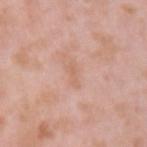| feature | finding |
|---|---|
| workup | total-body-photography surveillance lesion; no biopsy |
| location | the left upper arm |
| patient | male, about 55 years old |
| image source | ~15 mm tile from a whole-body skin photo |
| image-analysis metrics | an area of roughly 1.5 mm² and an outline eccentricity of about 0.95 (0 = round, 1 = elongated); border irregularity of about 6 on a 0–10 scale and peripheral color asymmetry of about 0 |
| size | ≈2.5 mm |